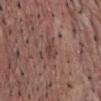Findings:
- biopsy status · imaged on a skin check; not biopsied
- anatomic site · the chest
- subject · male, in their mid-50s
- tile lighting · white-light
- imaging modality · total-body-photography crop, ~15 mm field of view